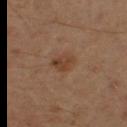Measured at roughly 3 mm in maximum diameter.
Located on the left upper arm.
A 15 mm close-up tile from a total-body photography series done for melanoma screening.
The subject is a male approximately 45 years of age.
An algorithmic analysis of the crop reported a lesion area of about 4.5 mm² and a shape-asymmetry score of about 0.2 (0 = symmetric).
This is a cross-polarized tile.